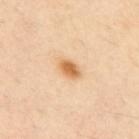Case summary:
– workup · total-body-photography surveillance lesion; no biopsy
– automated metrics · a border-irregularity index near 2/10, a color-variation rating of about 2.5/10, and radial color variation of about 1; a classifier nevus-likeness of about 95/100 and a lesion-detection confidence of about 100/100
– illumination · cross-polarized illumination
– body site · the upper back
– diameter · about 3 mm
– acquisition · ~15 mm crop, total-body skin-cancer survey
– patient · male, approximately 55 years of age